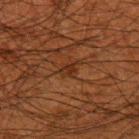Q: Was a biopsy performed?
A: catalogued during a skin exam; not biopsied
Q: What is the imaging modality?
A: 15 mm crop, total-body photography
Q: What is the lesion's diameter?
A: ~3 mm (longest diameter)
Q: How was the tile lit?
A: cross-polarized
Q: What is the anatomic site?
A: the arm
Q: What are the patient's age and sex?
A: male, aged approximately 50
Q: What did automated image analysis measure?
A: a mean CIELAB color near L≈24 a*≈18 b*≈26, about 6 CIELAB-L* units darker than the surrounding skin, and a lesion-to-skin contrast of about 6.5 (normalized; higher = more distinct); a classifier nevus-likeness of about 0/100